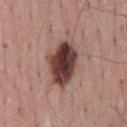workup: imaged on a skin check; not biopsied
imaging modality: ~15 mm tile from a whole-body skin photo
subject: male, aged approximately 50
anatomic site: the back